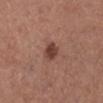The lesion was tiled from a total-body skin photograph and was not biopsied. Approximately 2.5 mm at its widest. The lesion is on the left lower leg. The patient is a female approximately 60 years of age. This is a white-light tile. An algorithmic analysis of the crop reported an area of roughly 5 mm² and an outline eccentricity of about 0.55 (0 = round, 1 = elongated). The software also gave a lesion color around L≈42 a*≈22 b*≈25 in CIELAB and a lesion-to-skin contrast of about 9 (normalized; higher = more distinct). And it measured a border-irregularity index near 2/10, internal color variation of about 2.5 on a 0–10 scale, and peripheral color asymmetry of about 1. A close-up tile cropped from a whole-body skin photograph, about 15 mm across.